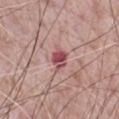Findings:
* biopsy status: imaged on a skin check; not biopsied
* illumination: white-light
* TBP lesion metrics: a mean CIELAB color near L≈50 a*≈30 b*≈19, a lesion–skin lightness drop of about 14, and a lesion-to-skin contrast of about 10 (normalized; higher = more distinct); border irregularity of about 3 on a 0–10 scale; a nevus-likeness score of about 5/100 and a detector confidence of about 100 out of 100 that the crop contains a lesion
* image source: 15 mm crop, total-body photography
* size: ≈3 mm
* patient: male, approximately 70 years of age
* body site: the chest